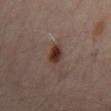The lesion is on the mid back.
This image is a 15 mm lesion crop taken from a total-body photograph.
The subject is roughly 55 years of age.